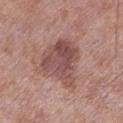No biopsy was performed on this lesion — it was imaged during a full skin examination and was not determined to be concerning. On the left lower leg. The subject is a male aged around 55. The total-body-photography lesion software estimated a border-irregularity index near 4/10, a color-variation rating of about 5/10, and radial color variation of about 1.5. A lesion tile, about 15 mm wide, cut from a 3D total-body photograph. The tile uses white-light illumination.